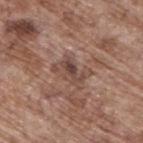biopsy_status: not biopsied; imaged during a skin examination
lesion_size:
  long_diameter_mm_approx: 4.0
automated_metrics:
  border_irregularity_0_10: 5.5
lighting: white-light
patient:
  sex: male
  age_approx: 70
site: upper back
image:
  source: total-body photography crop
  field_of_view_mm: 15